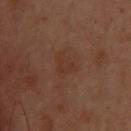biopsy status = catalogued during a skin exam; not biopsied
site = the head or neck
image-analysis metrics = a footprint of about 5.5 mm²; a classifier nevus-likeness of about 0/100 and lesion-presence confidence of about 100/100
illumination = cross-polarized illumination
subject = female, about 55 years old
diameter = about 3 mm
image = 15 mm crop, total-body photography